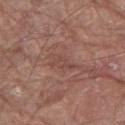Located on the left lower leg. A close-up tile cropped from a whole-body skin photograph, about 15 mm across. Longest diameter approximately 3.5 mm. A male subject aged around 80. This is a white-light tile.